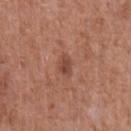This lesion was catalogued during total-body skin photography and was not selected for biopsy. Located on the chest. A 15 mm crop from a total-body photograph taken for skin-cancer surveillance. The patient is a male aged 63–67.